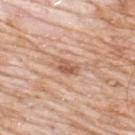biopsy_status: not biopsied; imaged during a skin examination
site: back
image:
  source: total-body photography crop
  field_of_view_mm: 15
patient:
  sex: male
  age_approx: 80
lesion_size:
  long_diameter_mm_approx: 2.5